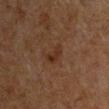The lesion was photographed on a routine skin check and not biopsied; there is no pathology result. Imaged with cross-polarized lighting. The lesion is on the chest. A 15 mm close-up tile from a total-body photography series done for melanoma screening. A male patient, aged 58 to 62. The total-body-photography lesion software estimated an average lesion color of about L≈22 a*≈14 b*≈22 (CIELAB), about 5 CIELAB-L* units darker than the surrounding skin, and a normalized border contrast of about 6.5. The analysis additionally found border irregularity of about 4.5 on a 0–10 scale, a within-lesion color-variation index near 2/10, and a peripheral color-asymmetry measure near 0.5. The software also gave a detector confidence of about 100 out of 100 that the crop contains a lesion. Approximately 3 mm at its widest.